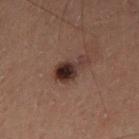follow-up: imaged on a skin check; not biopsied
lesion size: about 3.5 mm
lighting: cross-polarized illumination
site: the left thigh
subject: male, aged around 60
image-analysis metrics: internal color variation of about 6 on a 0–10 scale and radial color variation of about 2; an automated nevus-likeness rating near 75 out of 100 and a detector confidence of about 100 out of 100 that the crop contains a lesion
acquisition: 15 mm crop, total-body photography